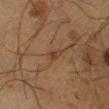Clinical impression: The lesion was tiled from a total-body skin photograph and was not biopsied. Clinical summary: The lesion is on the right lower leg. A male patient, in their 60s. Imaged with cross-polarized lighting. The recorded lesion diameter is about 2.5 mm. Cropped from a whole-body photographic skin survey; the tile spans about 15 mm.